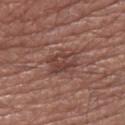This lesion was catalogued during total-body skin photography and was not selected for biopsy.
A male patient, aged around 75.
Captured under white-light illumination.
The recorded lesion diameter is about 3.5 mm.
The total-body-photography lesion software estimated a mean CIELAB color near L≈42 a*≈21 b*≈23, about 8 CIELAB-L* units darker than the surrounding skin, and a lesion-to-skin contrast of about 6.5 (normalized; higher = more distinct). And it measured border irregularity of about 3 on a 0–10 scale, internal color variation of about 4.5 on a 0–10 scale, and radial color variation of about 1.5. The analysis additionally found an automated nevus-likeness rating near 0 out of 100 and a lesion-detection confidence of about 95/100.
A close-up tile cropped from a whole-body skin photograph, about 15 mm across.
The lesion is located on the leg.